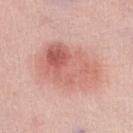A female patient aged approximately 45. Automated image analysis of the tile measured a lesion area of about 28 mm² and an outline eccentricity of about 0.8 (0 = round, 1 = elongated). Located on the right lower leg. Measured at roughly 7.5 mm in maximum diameter. Captured under white-light illumination. Cropped from a whole-body photographic skin survey; the tile spans about 15 mm. Histopathology of the biopsied lesion showed an indeterminate (borderline) lesion: atypical melanocytic neoplasm.